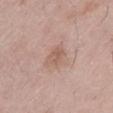Assessment: Captured during whole-body skin photography for melanoma surveillance; the lesion was not biopsied. Image and clinical context: The lesion is on the abdomen. This is a white-light tile. A roughly 15 mm field-of-view crop from a total-body skin photograph. Automated tile analysis of the lesion measured a classifier nevus-likeness of about 0/100. The lesion's longest dimension is about 3 mm. A male patient, aged 48–52.